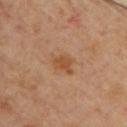<lesion>
  <biopsy_status>not biopsied; imaged during a skin examination</biopsy_status>
  <site>upper back</site>
  <patient>
    <sex>female</sex>
    <age_approx>60</age_approx>
  </patient>
  <automated_metrics>
    <border_irregularity_0_10>3.0</border_irregularity_0_10>
    <color_variation_0_10>2.0</color_variation_0_10>
    <peripheral_color_asymmetry>0.5</peripheral_color_asymmetry>
    <nevus_likeness_0_100>40</nevus_likeness_0_100>
    <lesion_detection_confidence_0_100>100</lesion_detection_confidence_0_100>
  </automated_metrics>
  <image>
    <source>total-body photography crop</source>
    <field_of_view_mm>15</field_of_view_mm>
  </image>
</lesion>